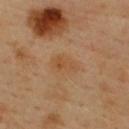No biopsy was performed on this lesion — it was imaged during a full skin examination and was not determined to be concerning.
The lesion's longest dimension is about 3.5 mm.
A lesion tile, about 15 mm wide, cut from a 3D total-body photograph.
A male subject about 40 years old.
Automated image analysis of the tile measured a within-lesion color-variation index near 3/10 and a peripheral color-asymmetry measure near 1.
The lesion is located on the upper back.